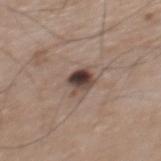Longest diameter approximately 2.5 mm. An algorithmic analysis of the crop reported a lesion color around L≈42 a*≈15 b*≈21 in CIELAB, a lesion–skin lightness drop of about 16, and a normalized lesion–skin contrast near 12. The lesion is on the mid back. A male patient, approximately 75 years of age. A 15 mm crop from a total-body photograph taken for skin-cancer surveillance.